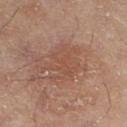Captured during whole-body skin photography for melanoma surveillance; the lesion was not biopsied. The patient is a female aged 58–62. A roughly 15 mm field-of-view crop from a total-body skin photograph. This is a cross-polarized tile. On the left lower leg.